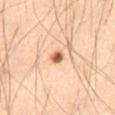{
  "patient": {
    "sex": "male",
    "age_approx": 65
  },
  "image": {
    "source": "total-body photography crop",
    "field_of_view_mm": 15
  },
  "lighting": "cross-polarized",
  "automated_metrics": {
    "area_mm2_approx": 2.5,
    "cielab_L": 59,
    "cielab_a": 23,
    "cielab_b": 35,
    "vs_skin_darker_L": 16.0,
    "vs_skin_contrast_norm": 10.0,
    "nevus_likeness_0_100": 100
  },
  "lesion_size": {
    "long_diameter_mm_approx": 2.0
  },
  "site": "front of the torso"
}